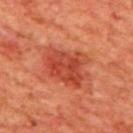Part of a total-body skin-imaging series; this lesion was reviewed on a skin check and was not flagged for biopsy. About 5.5 mm across. The lesion is located on the mid back. A close-up tile cropped from a whole-body skin photograph, about 15 mm across. Captured under cross-polarized illumination. A male subject about 65 years old.